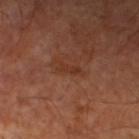Clinical impression: Imaged during a routine full-body skin examination; the lesion was not biopsied and no histopathology is available. Acquisition and patient details: The recorded lesion diameter is about 2.5 mm. The lesion is on the left lower leg. Captured under cross-polarized illumination. A male subject approximately 60 years of age. A lesion tile, about 15 mm wide, cut from a 3D total-body photograph.